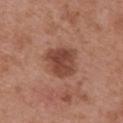– follow-up — total-body-photography surveillance lesion; no biopsy
– size — ~4.5 mm (longest diameter)
– site — the upper back
– automated lesion analysis — a footprint of about 12 mm², an eccentricity of roughly 0.4, and a shape-asymmetry score of about 0.25 (0 = symmetric)
– patient — female, aged 38–42
– imaging modality — total-body-photography crop, ~15 mm field of view